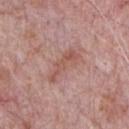notes = catalogued during a skin exam; not biopsied
lesion size = ~5 mm (longest diameter)
imaging modality = ~15 mm crop, total-body skin-cancer survey
image-analysis metrics = an area of roughly 6 mm², an eccentricity of roughly 0.95, and a symmetry-axis asymmetry near 0.5
patient = male, in their 70s
site = the chest